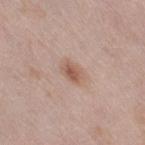Q: Is there a histopathology result?
A: imaged on a skin check; not biopsied
Q: Where on the body is the lesion?
A: the right thigh
Q: How large is the lesion?
A: ≈3 mm
Q: How was this image acquired?
A: ~15 mm tile from a whole-body skin photo
Q: What are the patient's age and sex?
A: female, aged 38 to 42
Q: What lighting was used for the tile?
A: white-light illumination